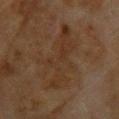Recorded during total-body skin imaging; not selected for excision or biopsy. Measured at roughly 9 mm in maximum diameter. A 15 mm close-up tile from a total-body photography series done for melanoma screening. From the head or neck. A female patient about 60 years old.